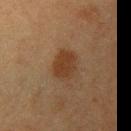workup: catalogued during a skin exam; not biopsied | anatomic site: the left upper arm | automated metrics: an area of roughly 8.5 mm², an eccentricity of roughly 0.55, and two-axis asymmetry of about 0.15; a mean CIELAB color near L≈30 a*≈16 b*≈27, about 7 CIELAB-L* units darker than the surrounding skin, and a normalized border contrast of about 8; a within-lesion color-variation index near 2.5/10 and radial color variation of about 1; lesion-presence confidence of about 100/100 | subject: female, in their mid- to late 50s | image: total-body-photography crop, ~15 mm field of view | lesion size: ~3.5 mm (longest diameter).